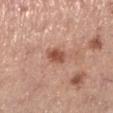Q: Is there a histopathology result?
A: imaged on a skin check; not biopsied
Q: Patient demographics?
A: female, roughly 65 years of age
Q: Automated lesion metrics?
A: an average lesion color of about L≈52 a*≈24 b*≈30 (CIELAB), a lesion–skin lightness drop of about 13, and a lesion-to-skin contrast of about 9 (normalized; higher = more distinct); a nevus-likeness score of about 85/100 and a lesion-detection confidence of about 100/100
Q: What is the lesion's diameter?
A: ~3 mm (longest diameter)
Q: What lighting was used for the tile?
A: white-light
Q: What is the anatomic site?
A: the left lower leg
Q: What kind of image is this?
A: ~15 mm crop, total-body skin-cancer survey